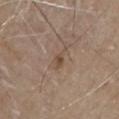Imaged during a routine full-body skin examination; the lesion was not biopsied and no histopathology is available. The lesion is on the chest. A male patient, approximately 80 years of age. Measured at roughly 3 mm in maximum diameter. This is a white-light tile. A close-up tile cropped from a whole-body skin photograph, about 15 mm across.